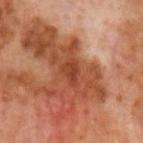Q: Was a biopsy performed?
A: total-body-photography surveillance lesion; no biopsy
Q: Lesion location?
A: the head or neck
Q: How was this image acquired?
A: total-body-photography crop, ~15 mm field of view
Q: What did automated image analysis measure?
A: a footprint of about 4 mm² and a shape-asymmetry score of about 0.3 (0 = symmetric); an average lesion color of about L≈42 a*≈29 b*≈34 (CIELAB) and a lesion–skin lightness drop of about 8
Q: How was the tile lit?
A: cross-polarized
Q: What is the lesion's diameter?
A: ≈3 mm
Q: Patient demographics?
A: male, in their 60s